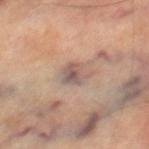Impression: Captured during whole-body skin photography for melanoma surveillance; the lesion was not biopsied. Acquisition and patient details: A male subject, approximately 70 years of age. Cropped from a total-body skin-imaging series; the visible field is about 15 mm. The total-body-photography lesion software estimated a lesion area of about 5.5 mm², a shape eccentricity near 0.45, and two-axis asymmetry of about 0.25. And it measured border irregularity of about 3 on a 0–10 scale, a color-variation rating of about 5.5/10, and radial color variation of about 2. The analysis additionally found an automated nevus-likeness rating near 0 out of 100 and a lesion-detection confidence of about 60/100. The recorded lesion diameter is about 3 mm. The lesion is located on the right thigh.